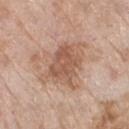Findings:
- biopsy status: no biopsy performed (imaged during a skin exam)
- automated metrics: an area of roughly 21 mm², a shape eccentricity near 0.65, and a shape-asymmetry score of about 0.35 (0 = symmetric); a color-variation rating of about 4/10 and radial color variation of about 1.5; a classifier nevus-likeness of about 10/100 and a detector confidence of about 100 out of 100 that the crop contains a lesion
- subject: female, aged approximately 70
- diameter: ~6 mm (longest diameter)
- site: the left lower leg
- acquisition: ~15 mm crop, total-body skin-cancer survey
- lighting: white-light illumination